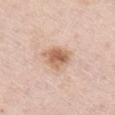notes: imaged on a skin check; not biopsied
lighting: white-light illumination
diameter: ~3.5 mm (longest diameter)
automated lesion analysis: a footprint of about 8 mm², an outline eccentricity of about 0.55 (0 = round, 1 = elongated), and a symmetry-axis asymmetry near 0.25; a lesion color around L≈63 a*≈20 b*≈32 in CIELAB and a normalized border contrast of about 8.5; a border-irregularity index near 2.5/10, a color-variation rating of about 5.5/10, and peripheral color asymmetry of about 2
patient: female, in their mid- to late 60s
image source: 15 mm crop, total-body photography
body site: the chest